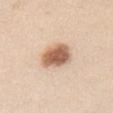<case>
<biopsy_status>not biopsied; imaged during a skin examination</biopsy_status>
<image>
  <source>total-body photography crop</source>
  <field_of_view_mm>15</field_of_view_mm>
</image>
<site>chest</site>
<lesion_size>
  <long_diameter_mm_approx>4.0</long_diameter_mm_approx>
</lesion_size>
<patient>
  <sex>female</sex>
  <age_approx>40</age_approx>
</patient>
</case>